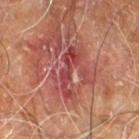A male patient aged approximately 60.
A 15 mm close-up tile from a total-body photography series done for melanoma screening.
Located on the right leg.
Imaged with cross-polarized lighting.
Approximately 6 mm at its widest.